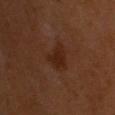Case summary:
– notes · total-body-photography surveillance lesion; no biopsy
– subject · male, aged 58 to 62
– acquisition · 15 mm crop, total-body photography
– location · the head or neck